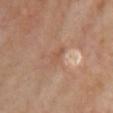- biopsy status · no biopsy performed (imaged during a skin exam)
- lesion diameter · about 2.5 mm
- imaging modality · total-body-photography crop, ~15 mm field of view
- patient · female, aged 58–62
- site · the front of the torso